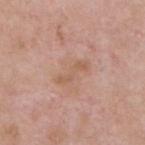Captured during whole-body skin photography for melanoma surveillance; the lesion was not biopsied. A male subject, about 55 years old. Automated tile analysis of the lesion measured a border-irregularity index near 6.5/10, internal color variation of about 0 on a 0–10 scale, and peripheral color asymmetry of about 0. It also reported a nevus-likeness score of about 0/100 and a lesion-detection confidence of about 100/100. This is a white-light tile. On the chest. A lesion tile, about 15 mm wide, cut from a 3D total-body photograph. Approximately 4 mm at its widest.